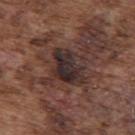Assessment: The lesion was photographed on a routine skin check and not biopsied; there is no pathology result. Context: A lesion tile, about 15 mm wide, cut from a 3D total-body photograph. A male subject, in their mid-70s. About 5 mm across. Captured under white-light illumination. Located on the upper back. Automated tile analysis of the lesion measured a lesion area of about 12 mm² and a shape-asymmetry score of about 0.35 (0 = symmetric). It also reported a border-irregularity index near 3.5/10 and a peripheral color-asymmetry measure near 2. And it measured a nevus-likeness score of about 0/100 and a detector confidence of about 80 out of 100 that the crop contains a lesion.